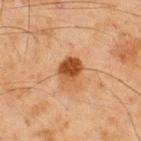Impression:
This lesion was catalogued during total-body skin photography and was not selected for biopsy.
Context:
The subject is a male roughly 45 years of age. A lesion tile, about 15 mm wide, cut from a 3D total-body photograph. This is a cross-polarized tile. The total-body-photography lesion software estimated a footprint of about 7 mm², an eccentricity of roughly 0.55, and a shape-asymmetry score of about 0.25 (0 = symmetric). Located on the back.